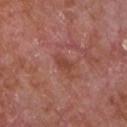Assessment:
The lesion was tiled from a total-body skin photograph and was not biopsied.
Acquisition and patient details:
This image is a 15 mm lesion crop taken from a total-body photograph. Longest diameter approximately 2.5 mm. A male patient, about 65 years old. On the chest. The lesion-visualizer software estimated an average lesion color of about L≈44 a*≈26 b*≈28 (CIELAB), roughly 7 lightness units darker than nearby skin, and a normalized border contrast of about 6.5. The analysis additionally found a classifier nevus-likeness of about 0/100 and a detector confidence of about 100 out of 100 that the crop contains a lesion. The tile uses white-light illumination.